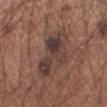notes = imaged on a skin check; not biopsied | tile lighting = white-light | patient = male, approximately 55 years of age | size = ≈6.5 mm | site = the left forearm | imaging modality = total-body-photography crop, ~15 mm field of view.